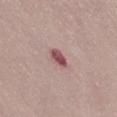The lesion was tiled from a total-body skin photograph and was not biopsied.
The tile uses white-light illumination.
A 15 mm crop from a total-body photograph taken for skin-cancer surveillance.
The total-body-photography lesion software estimated an eccentricity of roughly 0.75. The analysis additionally found about 14 CIELAB-L* units darker than the surrounding skin and a normalized border contrast of about 9.5.
Approximately 2.5 mm at its widest.
A female subject about 50 years old.
The lesion is on the abdomen.